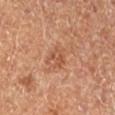Q: Was this lesion biopsied?
A: total-body-photography surveillance lesion; no biopsy
Q: Illumination type?
A: cross-polarized illumination
Q: Where on the body is the lesion?
A: the left lower leg
Q: How large is the lesion?
A: ≈2.5 mm
Q: What kind of image is this?
A: 15 mm crop, total-body photography
Q: Who is the patient?
A: female